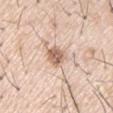Captured during whole-body skin photography for melanoma surveillance; the lesion was not biopsied. The tile uses white-light illumination. Automated tile analysis of the lesion measured a lesion area of about 5 mm². The software also gave about 14 CIELAB-L* units darker than the surrounding skin and a normalized lesion–skin contrast near 8.5. It also reported radial color variation of about 1. And it measured a nevus-likeness score of about 90/100 and a lesion-detection confidence of about 100/100. A close-up tile cropped from a whole-body skin photograph, about 15 mm across. A male patient, in their mid- to late 50s.